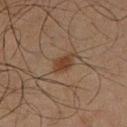<lesion>
  <biopsy_status>not biopsied; imaged during a skin examination</biopsy_status>
  <lesion_size>
    <long_diameter_mm_approx>3.0</long_diameter_mm_approx>
  </lesion_size>
  <patient>
    <sex>male</sex>
    <age_approx>65</age_approx>
  </patient>
  <site>left lower leg</site>
  <image>
    <source>total-body photography crop</source>
    <field_of_view_mm>15</field_of_view_mm>
  </image>
  <automated_metrics>
    <area_mm2_approx>4.5</area_mm2_approx>
    <eccentricity>0.75</eccentricity>
    <shape_asymmetry>0.25</shape_asymmetry>
  </automated_metrics>
</lesion>